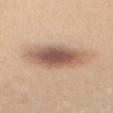follow-up=catalogued during a skin exam; not biopsied
acquisition=total-body-photography crop, ~15 mm field of view
patient=female, aged around 30
body site=the mid back
lesion size=about 7 mm